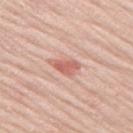The lesion was tiled from a total-body skin photograph and was not biopsied.
A male patient, approximately 70 years of age.
A close-up tile cropped from a whole-body skin photograph, about 15 mm across.
Located on the left thigh.